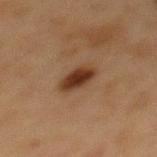{"biopsy_status": "not biopsied; imaged during a skin examination", "lesion_size": {"long_diameter_mm_approx": 3.5}, "lighting": "cross-polarized", "image": {"source": "total-body photography crop", "field_of_view_mm": 15}, "patient": {"sex": "female", "age_approx": 40}, "automated_metrics": {"area_mm2_approx": 7.0, "eccentricity": 0.75, "border_irregularity_0_10": 1.5, "color_variation_0_10": 3.5, "peripheral_color_asymmetry": 1.0}, "site": "mid back"}